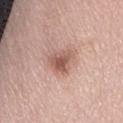Q: Was this lesion biopsied?
A: catalogued during a skin exam; not biopsied
Q: Who is the patient?
A: female, in their 60s
Q: Illumination type?
A: white-light illumination
Q: What is the lesion's diameter?
A: ≈3.5 mm
Q: Automated lesion metrics?
A: an area of roughly 7.5 mm²; a lesion color around L≈57 a*≈21 b*≈25 in CIELAB, about 12 CIELAB-L* units darker than the surrounding skin, and a normalized border contrast of about 7.5; internal color variation of about 4.5 on a 0–10 scale and radial color variation of about 1.5
Q: How was this image acquired?
A: ~15 mm tile from a whole-body skin photo
Q: Lesion location?
A: the front of the torso